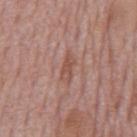Case summary:
- follow-up · no biopsy performed (imaged during a skin exam)
- patient · male, about 75 years old
- image-analysis metrics · a footprint of about 3.5 mm², an eccentricity of roughly 0.9, and a symmetry-axis asymmetry near 0.25; a lesion color around L≈51 a*≈23 b*≈26 in CIELAB and a normalized border contrast of about 6.5; border irregularity of about 3 on a 0–10 scale, internal color variation of about 1 on a 0–10 scale, and radial color variation of about 0; an automated nevus-likeness rating near 0 out of 100
- location · the back
- tile lighting · white-light
- lesion diameter · about 3 mm
- acquisition · ~15 mm crop, total-body skin-cancer survey